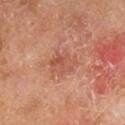Q: Was this lesion biopsied?
A: imaged on a skin check; not biopsied
Q: What kind of image is this?
A: ~15 mm tile from a whole-body skin photo
Q: Patient demographics?
A: male, aged around 65
Q: What is the anatomic site?
A: the right lower leg
Q: How large is the lesion?
A: ~4 mm (longest diameter)
Q: What lighting was used for the tile?
A: cross-polarized illumination